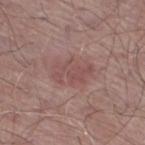Recorded during total-body skin imaging; not selected for excision or biopsy.
Measured at roughly 4.5 mm in maximum diameter.
A 15 mm close-up tile from a total-body photography series done for melanoma screening.
Located on the right thigh.
A male patient aged approximately 65.